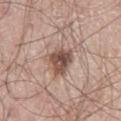Image and clinical context: The lesion is located on the right thigh. The tile uses white-light illumination. A male subject approximately 55 years of age. The total-body-photography lesion software estimated a border-irregularity index near 3.5/10, a color-variation rating of about 6/10, and radial color variation of about 2. Cropped from a whole-body photographic skin survey; the tile spans about 15 mm.